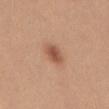No biopsy was performed on this lesion — it was imaged during a full skin examination and was not determined to be concerning. Located on the abdomen. The subject is a female aged 23 to 27. A lesion tile, about 15 mm wide, cut from a 3D total-body photograph.